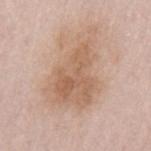{
  "lighting": "white-light",
  "image": {
    "source": "total-body photography crop",
    "field_of_view_mm": 15
  },
  "site": "left upper arm",
  "lesion_size": {
    "long_diameter_mm_approx": 9.0
  },
  "automated_metrics": {
    "eccentricity": 0.8
  },
  "patient": {
    "sex": "male",
    "age_approx": 65
  }
}